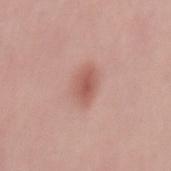workup: no biopsy performed (imaged during a skin exam) | diameter: about 3.5 mm | automated metrics: a lesion area of about 6 mm² and a shape-asymmetry score of about 0.25 (0 = symmetric); a border-irregularity rating of about 2.5/10, a color-variation rating of about 2.5/10, and peripheral color asymmetry of about 0.5; a nevus-likeness score of about 95/100 and a lesion-detection confidence of about 100/100 | subject: male, in their 30s | location: the mid back | illumination: white-light illumination | image source: ~15 mm crop, total-body skin-cancer survey.